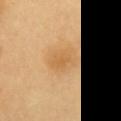Cropped from a whole-body photographic skin survey; the tile spans about 15 mm.
The lesion is on the chest.
Longest diameter approximately 2.5 mm.
A female patient aged around 60.
This is a cross-polarized tile.